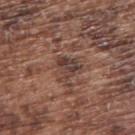Q: Automated lesion metrics?
A: a footprint of about 6 mm²; an average lesion color of about L≈40 a*≈18 b*≈22 (CIELAB), about 9 CIELAB-L* units darker than the surrounding skin, and a normalized lesion–skin contrast near 8.5; a nevus-likeness score of about 0/100 and a lesion-detection confidence of about 95/100
Q: Lesion location?
A: the right upper arm
Q: What kind of image is this?
A: total-body-photography crop, ~15 mm field of view
Q: Who is the patient?
A: male, in their mid-70s
Q: Lesion size?
A: ≈3 mm